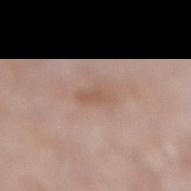No biopsy was performed on this lesion — it was imaged during a full skin examination and was not determined to be concerning. The tile uses white-light illumination. From the right lower leg. About 3 mm across. A close-up tile cropped from a whole-body skin photograph, about 15 mm across. A female subject aged 73 to 77.